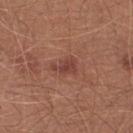Clinical impression:
Captured during whole-body skin photography for melanoma surveillance; the lesion was not biopsied.
Background:
This image is a 15 mm lesion crop taken from a total-body photograph. Measured at roughly 3 mm in maximum diameter. From the right lower leg. The tile uses white-light illumination. A male patient approximately 65 years of age. The total-body-photography lesion software estimated an area of roughly 4 mm², a shape eccentricity near 0.8, and a symmetry-axis asymmetry near 0.4. It also reported a lesion–skin lightness drop of about 8 and a lesion-to-skin contrast of about 7 (normalized; higher = more distinct). The analysis additionally found a border-irregularity index near 4.5/10, a color-variation rating of about 1.5/10, and radial color variation of about 0.5. And it measured a detector confidence of about 100 out of 100 that the crop contains a lesion.